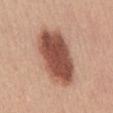The lesion was photographed on a routine skin check and not biopsied; there is no pathology result. A 15 mm close-up tile from a total-body photography series done for melanoma screening. Automated image analysis of the tile measured a lesion area of about 26 mm², an outline eccentricity of about 0.85 (0 = round, 1 = elongated), and a shape-asymmetry score of about 0.15 (0 = symmetric). It also reported a mean CIELAB color near L≈50 a*≈24 b*≈28, roughly 18 lightness units darker than nearby skin, and a lesion-to-skin contrast of about 12 (normalized; higher = more distinct). It also reported border irregularity of about 2 on a 0–10 scale, internal color variation of about 5 on a 0–10 scale, and radial color variation of about 2. It also reported a nevus-likeness score of about 100/100. About 8 mm across. A female patient, in their mid- to late 40s. Imaged with white-light lighting. The lesion is located on the abdomen.